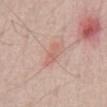Assessment:
This lesion was catalogued during total-body skin photography and was not selected for biopsy.
Acquisition and patient details:
Automated image analysis of the tile measured an area of roughly 3.5 mm², an eccentricity of roughly 0.9, and a shape-asymmetry score of about 0.35 (0 = symmetric). The software also gave a lesion color around L≈62 a*≈23 b*≈27 in CIELAB, about 7 CIELAB-L* units darker than the surrounding skin, and a normalized border contrast of about 5. The software also gave an automated nevus-likeness rating near 75 out of 100 and lesion-presence confidence of about 100/100. A 15 mm close-up extracted from a 3D total-body photography capture. The patient is a male aged around 70. The lesion is located on the chest.